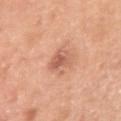Assessment: Recorded during total-body skin imaging; not selected for excision or biopsy. Clinical summary: Captured under white-light illumination. From the right upper arm. A 15 mm close-up tile from a total-body photography series done for melanoma screening. A male patient, about 50 years old. The lesion-visualizer software estimated border irregularity of about 3 on a 0–10 scale, internal color variation of about 3 on a 0–10 scale, and peripheral color asymmetry of about 1. And it measured a nevus-likeness score of about 45/100 and a lesion-detection confidence of about 100/100.